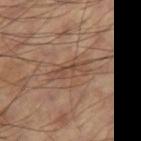Imaged during a routine full-body skin examination; the lesion was not biopsied and no histopathology is available.
A male subject aged 58 to 62.
The lesion is located on the right thigh.
This is a cross-polarized tile.
Measured at roughly 4.5 mm in maximum diameter.
Cropped from a whole-body photographic skin survey; the tile spans about 15 mm.
An algorithmic analysis of the crop reported a mean CIELAB color near L≈45 a*≈18 b*≈27, a lesion–skin lightness drop of about 7, and a normalized lesion–skin contrast near 5.5. It also reported border irregularity of about 5.5 on a 0–10 scale, internal color variation of about 3 on a 0–10 scale, and a peripheral color-asymmetry measure near 1. The software also gave an automated nevus-likeness rating near 0 out of 100 and a lesion-detection confidence of about 70/100.